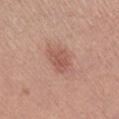<lesion>
  <biopsy_status>not biopsied; imaged during a skin examination</biopsy_status>
  <site>leg</site>
  <automated_metrics>
    <area_mm2_approx>6.5</area_mm2_approx>
    <color_variation_0_10>3.0</color_variation_0_10>
    <peripheral_color_asymmetry>1.0</peripheral_color_asymmetry>
    <nevus_likeness_0_100>75</nevus_likeness_0_100>
    <lesion_detection_confidence_0_100>100</lesion_detection_confidence_0_100>
  </automated_metrics>
  <lighting>white-light</lighting>
  <patient>
    <sex>female</sex>
    <age_approx>55</age_approx>
  </patient>
  <image>
    <source>total-body photography crop</source>
    <field_of_view_mm>15</field_of_view_mm>
  </image>
</lesion>